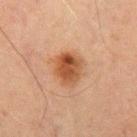Assessment: No biopsy was performed on this lesion — it was imaged during a full skin examination and was not determined to be concerning. Image and clinical context: The lesion-visualizer software estimated a mean CIELAB color near L≈42 a*≈21 b*≈31, a lesion–skin lightness drop of about 11, and a normalized lesion–skin contrast near 9.5. The analysis additionally found a within-lesion color-variation index near 6/10. It also reported a classifier nevus-likeness of about 95/100. The lesion is on the left thigh. A roughly 15 mm field-of-view crop from a total-body skin photograph. The patient is a male approximately 70 years of age. Longest diameter approximately 3.5 mm.